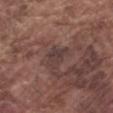Findings:
- notes: catalogued during a skin exam; not biopsied
- site: the left forearm
- lesion diameter: ≈3.5 mm
- imaging modality: total-body-photography crop, ~15 mm field of view
- patient: male, aged 73–77
- lighting: white-light illumination